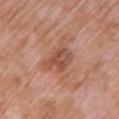| key | value |
|---|---|
| workup | total-body-photography surveillance lesion; no biopsy |
| lesion diameter | about 4 mm |
| acquisition | total-body-photography crop, ~15 mm field of view |
| anatomic site | the arm |
| subject | female, in their 70s |
| lighting | white-light |